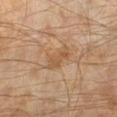Imaged during a routine full-body skin examination; the lesion was not biopsied and no histopathology is available.
A close-up tile cropped from a whole-body skin photograph, about 15 mm across.
The tile uses cross-polarized illumination.
From the left lower leg.
About 4 mm across.
The subject is a male aged around 60.